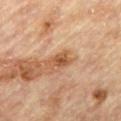Imaged during a routine full-body skin examination; the lesion was not biopsied and no histopathology is available.
Automated tile analysis of the lesion measured a footprint of about 4.5 mm².
The recorded lesion diameter is about 3 mm.
A roughly 15 mm field-of-view crop from a total-body skin photograph.
The lesion is on the mid back.
Imaged with cross-polarized lighting.
The patient is a male aged 83 or over.